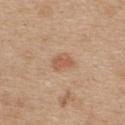No biopsy was performed on this lesion — it was imaged during a full skin examination and was not determined to be concerning. The patient is a female aged 43–47. On the upper back. A close-up tile cropped from a whole-body skin photograph, about 15 mm across. Imaged with white-light lighting.